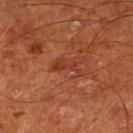Q: Was this lesion biopsied?
A: total-body-photography surveillance lesion; no biopsy
Q: Who is the patient?
A: male, in their mid-60s
Q: How was this image acquired?
A: ~15 mm tile from a whole-body skin photo
Q: How was the tile lit?
A: cross-polarized
Q: What is the anatomic site?
A: the leg